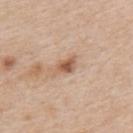workup: no biopsy performed (imaged during a skin exam) | site: the upper back | tile lighting: white-light illumination | imaging modality: ~15 mm tile from a whole-body skin photo | size: ≈3 mm | TBP lesion metrics: a footprint of about 4 mm², an eccentricity of roughly 0.8, and a symmetry-axis asymmetry near 0.3; a mean CIELAB color near L≈57 a*≈20 b*≈31, about 12 CIELAB-L* units darker than the surrounding skin, and a lesion-to-skin contrast of about 8 (normalized; higher = more distinct); a border-irregularity index near 3/10 and a peripheral color-asymmetry measure near 1.5 | subject: male, aged around 60.